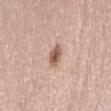biopsy status: no biopsy performed (imaged during a skin exam) | location: the back | TBP lesion metrics: a lesion area of about 4 mm², a shape eccentricity near 0.75, and a shape-asymmetry score of about 0.2 (0 = symmetric); a border-irregularity index near 2/10 and a within-lesion color-variation index near 3.5/10 | subject: female, roughly 50 years of age | lesion size: ≈3 mm | image source: 15 mm crop, total-body photography | illumination: white-light.